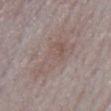notes: catalogued during a skin exam; not biopsied
anatomic site: the front of the torso
patient: male, roughly 65 years of age
acquisition: total-body-photography crop, ~15 mm field of view
TBP lesion metrics: a footprint of about 15 mm², a shape eccentricity near 0.85, and a shape-asymmetry score of about 0.5 (0 = symmetric); an average lesion color of about L≈54 a*≈14 b*≈20 (CIELAB) and about 6 CIELAB-L* units darker than the surrounding skin; border irregularity of about 6 on a 0–10 scale and internal color variation of about 4 on a 0–10 scale; an automated nevus-likeness rating near 5 out of 100 and a lesion-detection confidence of about 50/100
lesion diameter: ≈6.5 mm